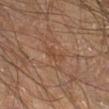biopsy status=total-body-photography surveillance lesion; no biopsy | patient=male, roughly 50 years of age | lesion size=≈3 mm | illumination=cross-polarized illumination | image source=~15 mm crop, total-body skin-cancer survey | anatomic site=the leg.